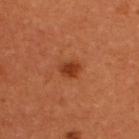Part of a total-body skin-imaging series; this lesion was reviewed on a skin check and was not flagged for biopsy. The tile uses cross-polarized illumination. Longest diameter approximately 2.5 mm. Automated image analysis of the tile measured a lesion area of about 4.5 mm² and a shape-asymmetry score of about 0.2 (0 = symmetric). It also reported an average lesion color of about L≈40 a*≈30 b*≈38 (CIELAB) and a lesion-to-skin contrast of about 8 (normalized; higher = more distinct). Cropped from a total-body skin-imaging series; the visible field is about 15 mm. The subject is a female aged around 50.